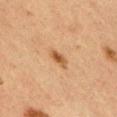Clinical impression:
The lesion was photographed on a routine skin check and not biopsied; there is no pathology result.
Acquisition and patient details:
The lesion is on the chest. The patient is a male approximately 35 years of age. This image is a 15 mm lesion crop taken from a total-body photograph. The tile uses cross-polarized illumination.